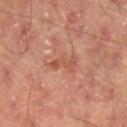Part of a total-body skin-imaging series; this lesion was reviewed on a skin check and was not flagged for biopsy. A 15 mm crop from a total-body photograph taken for skin-cancer surveillance. From the right lower leg. A male subject, in their mid- to late 60s. Automated tile analysis of the lesion measured a footprint of about 5 mm², an outline eccentricity of about 0.9 (0 = round, 1 = elongated), and two-axis asymmetry of about 0.45. The software also gave a mean CIELAB color near L≈52 a*≈26 b*≈31 and a lesion–skin lightness drop of about 7.